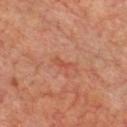biopsy status: catalogued during a skin exam; not biopsied | subject: male, approximately 70 years of age | imaging modality: ~15 mm tile from a whole-body skin photo | site: the chest | illumination: cross-polarized | lesion diameter: ~3 mm (longest diameter).